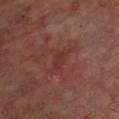  biopsy_status: not biopsied; imaged during a skin examination
  lighting: cross-polarized
  patient:
    age_approx: 55
  lesion_size:
    long_diameter_mm_approx: 2.5
  image:
    source: total-body photography crop
    field_of_view_mm: 15
  site: chest